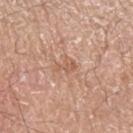The tile uses white-light illumination.
About 2.5 mm across.
A 15 mm close-up extracted from a 3D total-body photography capture.
The patient is a male about 60 years old.
Located on the left upper arm.